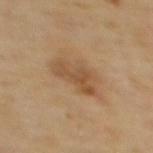biopsy status=imaged on a skin check; not biopsied
lesion size=about 5 mm
patient=female, aged 68–72
body site=the upper back
tile lighting=cross-polarized illumination
image=~15 mm tile from a whole-body skin photo